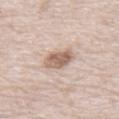notes: imaged on a skin check; not biopsied
subject: male, aged around 80
tile lighting: white-light illumination
imaging modality: 15 mm crop, total-body photography
body site: the abdomen
diameter: ~3 mm (longest diameter)